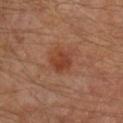Context: The lesion is on the right lower leg. A close-up tile cropped from a whole-body skin photograph, about 15 mm across. The subject is a male roughly 65 years of age.